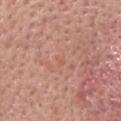Case summary:
* workup — total-body-photography surveillance lesion; no biopsy
* lighting — white-light illumination
* image — ~15 mm tile from a whole-body skin photo
* size — ~1.5 mm (longest diameter)
* automated metrics — a lesion color around L≈58 a*≈24 b*≈30 in CIELAB, a lesion–skin lightness drop of about 4, and a lesion-to-skin contrast of about 3.5 (normalized; higher = more distinct); a border-irregularity index near 2.5/10, a color-variation rating of about 0/10, and a peripheral color-asymmetry measure near 0
* subject — female, aged approximately 60
* body site — the head or neck